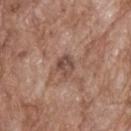image source: total-body-photography crop, ~15 mm field of view | patient: male, about 70 years old | anatomic site: the upper back.